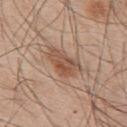Q: Is there a histopathology result?
A: total-body-photography surveillance lesion; no biopsy
Q: How was this image acquired?
A: 15 mm crop, total-body photography
Q: Patient demographics?
A: male, aged 53 to 57
Q: How large is the lesion?
A: ≈4.5 mm
Q: What is the anatomic site?
A: the upper back